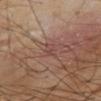<case>
<site>right upper arm</site>
<lesion_size>
  <long_diameter_mm_approx>2.5</long_diameter_mm_approx>
</lesion_size>
<image>
  <source>total-body photography crop</source>
  <field_of_view_mm>15</field_of_view_mm>
</image>
<lighting>cross-polarized</lighting>
<automated_metrics>
  <cielab_L>46</cielab_L>
  <cielab_a>20</cielab_a>
  <cielab_b>23</cielab_b>
  <vs_skin_darker_L>6.0</vs_skin_darker_L>
  <vs_skin_contrast_norm>5.0</vs_skin_contrast_norm>
  <border_irregularity_0_10>3.0</border_irregularity_0_10>
  <color_variation_0_10>1.0</color_variation_0_10>
  <peripheral_color_asymmetry>0.0</peripheral_color_asymmetry>
  <nevus_likeness_0_100>0</nevus_likeness_0_100>
</automated_metrics>
</case>